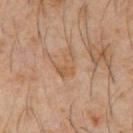biopsy status=no biopsy performed (imaged during a skin exam)
lighting=cross-polarized
size=about 3 mm
automated metrics=a footprint of about 4 mm² and a shape eccentricity near 0.75; a border-irregularity rating of about 6.5/10 and radial color variation of about 0.5; a nevus-likeness score of about 0/100 and a lesion-detection confidence of about 100/100
image=~15 mm crop, total-body skin-cancer survey
patient=male, aged around 60
site=the chest